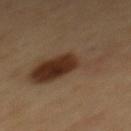  biopsy_status: not biopsied; imaged during a skin examination
  image:
    source: total-body photography crop
    field_of_view_mm: 15
  patient:
    sex: male
    age_approx: 50
  lighting: cross-polarized
  site: mid back